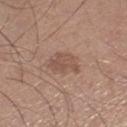Recorded during total-body skin imaging; not selected for excision or biopsy.
A male patient in their mid- to late 70s.
Cropped from a whole-body photographic skin survey; the tile spans about 15 mm.
Automated tile analysis of the lesion measured a footprint of about 7 mm² and a shape-asymmetry score of about 0.3 (0 = symmetric). The analysis additionally found a nevus-likeness score of about 20/100 and a detector confidence of about 100 out of 100 that the crop contains a lesion.
The lesion is located on the right thigh.
Measured at roughly 3.5 mm in maximum diameter.